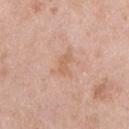{
  "biopsy_status": "not biopsied; imaged during a skin examination",
  "site": "upper back",
  "lighting": "white-light",
  "image": {
    "source": "total-body photography crop",
    "field_of_view_mm": 15
  },
  "lesion_size": {
    "long_diameter_mm_approx": 3.0
  },
  "patient": {
    "sex": "male",
    "age_approx": 25
  }
}